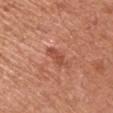Q: Is there a histopathology result?
A: imaged on a skin check; not biopsied